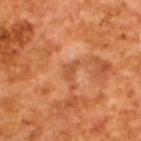Findings:
• illumination: cross-polarized
• patient: male, in their mid-60s
• image: total-body-photography crop, ~15 mm field of view
• size: about 2.5 mm